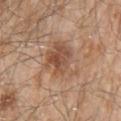Recorded during total-body skin imaging; not selected for excision or biopsy. The subject is a male aged approximately 80. A lesion tile, about 15 mm wide, cut from a 3D total-body photograph. Located on the left upper arm. About 5.5 mm across. This is a white-light tile. An algorithmic analysis of the crop reported a lesion area of about 19 mm², a shape eccentricity near 0.35, and a symmetry-axis asymmetry near 0.2.